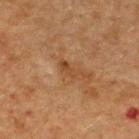Captured during whole-body skin photography for melanoma surveillance; the lesion was not biopsied.
A 15 mm close-up tile from a total-body photography series done for melanoma screening.
A male subject, about 50 years old.
The lesion is located on the upper back.